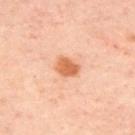Impression: Captured during whole-body skin photography for melanoma surveillance; the lesion was not biopsied. Context: The lesion is located on the back. A patient in their mid-50s. The lesion's longest dimension is about 3 mm. A 15 mm close-up tile from a total-body photography series done for melanoma screening. The lesion-visualizer software estimated a lesion area of about 5 mm², an outline eccentricity of about 0.6 (0 = round, 1 = elongated), and a shape-asymmetry score of about 0.25 (0 = symmetric). It also reported a lesion color around L≈64 a*≈28 b*≈40 in CIELAB. It also reported a border-irregularity index near 2/10 and a peripheral color-asymmetry measure near 1. This is a cross-polarized tile.